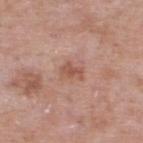Assessment: Captured during whole-body skin photography for melanoma surveillance; the lesion was not biopsied. Background: A male patient, approximately 55 years of age. A region of skin cropped from a whole-body photographic capture, roughly 15 mm wide. On the back. Automated image analysis of the tile measured an outline eccentricity of about 0.8 (0 = round, 1 = elongated). And it measured a lesion color around L≈54 a*≈23 b*≈29 in CIELAB and a lesion–skin lightness drop of about 9. The analysis additionally found a color-variation rating of about 2/10 and peripheral color asymmetry of about 0.5.